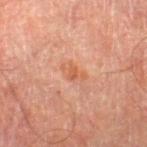Impression: Part of a total-body skin-imaging series; this lesion was reviewed on a skin check and was not flagged for biopsy. Image and clinical context: A 15 mm close-up extracted from a 3D total-body photography capture. The patient is a male aged 68 to 72. Approximately 3 mm at its widest. Captured under cross-polarized illumination. From the right thigh.